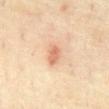| key | value |
|---|---|
| notes | imaged on a skin check; not biopsied |
| patient | male, aged 63–67 |
| image source | 15 mm crop, total-body photography |
| tile lighting | cross-polarized illumination |
| location | the front of the torso |
| size | ≈2.5 mm |